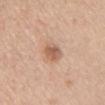{"biopsy_status": "not biopsied; imaged during a skin examination", "site": "chest", "lighting": "white-light", "image": {"source": "total-body photography crop", "field_of_view_mm": 15}, "lesion_size": {"long_diameter_mm_approx": 3.0}, "automated_metrics": {"border_irregularity_0_10": 1.5, "peripheral_color_asymmetry": 1.5}, "patient": {"sex": "female", "age_approx": 45}}